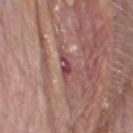notes = total-body-photography surveillance lesion; no biopsy
patient = male, about 65 years old
size = about 3 mm
lighting = white-light illumination
image = ~15 mm tile from a whole-body skin photo
site = the head or neck